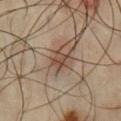{
  "biopsy_status": "not biopsied; imaged during a skin examination",
  "image": {
    "source": "total-body photography crop",
    "field_of_view_mm": 15
  },
  "patient": {
    "sex": "male",
    "age_approx": 65
  },
  "site": "front of the torso",
  "lesion_size": {
    "long_diameter_mm_approx": 2.5
  },
  "lighting": "cross-polarized"
}